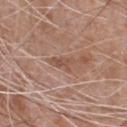  biopsy_status: not biopsied; imaged during a skin examination
  image:
    source: total-body photography crop
    field_of_view_mm: 15
  patient:
    sex: male
    age_approx: 65
  lesion_size:
    long_diameter_mm_approx: 3.0
  site: chest